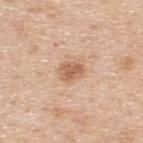<case>
<biopsy_status>not biopsied; imaged during a skin examination</biopsy_status>
</case>